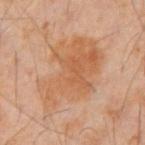notes = catalogued during a skin exam; not biopsied
patient = male, aged 58–62
site = the abdomen
automated lesion analysis = an area of roughly 38 mm², a shape eccentricity near 0.8, and two-axis asymmetry of about 0.3; a nevus-likeness score of about 0/100 and lesion-presence confidence of about 100/100
image = ~15 mm crop, total-body skin-cancer survey
lighting = cross-polarized